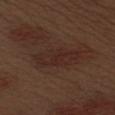A roughly 15 mm field-of-view crop from a total-body skin photograph.
The lesion is on the left upper arm.
The tile uses white-light illumination.
A male subject aged 68 to 72.
Automated tile analysis of the lesion measured border irregularity of about 4 on a 0–10 scale, a color-variation rating of about 2.5/10, and peripheral color asymmetry of about 1. And it measured an automated nevus-likeness rating near 0 out of 100 and a lesion-detection confidence of about 95/100.
Approximately 6.5 mm at its widest.